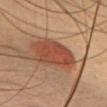No biopsy was performed on this lesion — it was imaged during a full skin examination and was not determined to be concerning.
The lesion is on the head or neck.
Measured at roughly 6 mm in maximum diameter.
This is a cross-polarized tile.
Cropped from a whole-body photographic skin survey; the tile spans about 15 mm.
An algorithmic analysis of the crop reported a lesion color around L≈37 a*≈21 b*≈26 in CIELAB, about 10 CIELAB-L* units darker than the surrounding skin, and a normalized border contrast of about 8.5. And it measured lesion-presence confidence of about 95/100.
A female subject, aged 38–42.